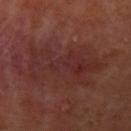Clinical impression: This lesion was catalogued during total-body skin photography and was not selected for biopsy. Background: On the left upper arm. This image is a 15 mm lesion crop taken from a total-body photograph. Imaged with cross-polarized lighting. A female patient, approximately 40 years of age. Approximately 9 mm at its widest.